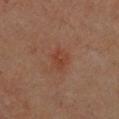• automated metrics · a lesion area of about 3 mm² and an outline eccentricity of about 0.85 (0 = round, 1 = elongated); a border-irregularity rating of about 3/10 and radial color variation of about 0.5
• tile lighting · cross-polarized
• patient · female, aged 63 to 67
• imaging modality · total-body-photography crop, ~15 mm field of view
• body site · the chest